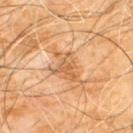Assessment: Captured during whole-body skin photography for melanoma surveillance; the lesion was not biopsied. Background: From the chest. A male subject in their 60s. Approximately 4 mm at its widest. A region of skin cropped from a whole-body photographic capture, roughly 15 mm wide. Captured under cross-polarized illumination.